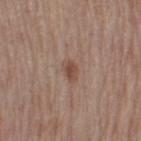{
  "biopsy_status": "not biopsied; imaged during a skin examination",
  "site": "left thigh",
  "patient": {
    "sex": "female",
    "age_approx": 40
  },
  "image": {
    "source": "total-body photography crop",
    "field_of_view_mm": 15
  }
}